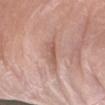Context: The lesion's longest dimension is about 3 mm. A 15 mm crop from a total-body photograph taken for skin-cancer surveillance. Located on the right forearm. This is a white-light tile. The subject is a male about 60 years old.